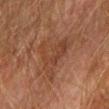biopsy status = total-body-photography surveillance lesion; no biopsy | imaging modality = total-body-photography crop, ~15 mm field of view | anatomic site = the right upper arm | patient = male, aged around 75.